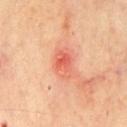Clinical impression:
The lesion was photographed on a routine skin check and not biopsied; there is no pathology result.
Image and clinical context:
Automated image analysis of the tile measured a lesion area of about 7.5 mm² and a symmetry-axis asymmetry near 0.2. And it measured a classifier nevus-likeness of about 0/100 and a detector confidence of about 100 out of 100 that the crop contains a lesion. A male subject, aged around 65. The lesion is located on the chest. About 3.5 mm across. A region of skin cropped from a whole-body photographic capture, roughly 15 mm wide. The tile uses cross-polarized illumination.